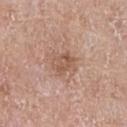{"biopsy_status": "not biopsied; imaged during a skin examination", "site": "chest", "image": {"source": "total-body photography crop", "field_of_view_mm": 15}, "patient": {"sex": "male", "age_approx": 70}, "lighting": "white-light", "lesion_size": {"long_diameter_mm_approx": 3.0}, "automated_metrics": {"cielab_L": 55, "cielab_a": 20, "cielab_b": 28, "vs_skin_darker_L": 8.0, "border_irregularity_0_10": 2.0, "peripheral_color_asymmetry": 1.5, "nevus_likeness_0_100": 5}}